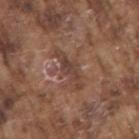This lesion was catalogued during total-body skin photography and was not selected for biopsy. The lesion is located on the arm. A male subject aged approximately 75. Automated tile analysis of the lesion measured a lesion color around L≈41 a*≈19 b*≈25 in CIELAB, a lesion–skin lightness drop of about 8, and a lesion-to-skin contrast of about 6.5 (normalized; higher = more distinct). The analysis additionally found a border-irregularity index near 6/10, internal color variation of about 2.5 on a 0–10 scale, and a peripheral color-asymmetry measure near 0.5. This is a white-light tile. Cropped from a whole-body photographic skin survey; the tile spans about 15 mm. Approximately 5.5 mm at its widest.